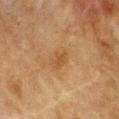notes — catalogued during a skin exam; not biopsied | illumination — cross-polarized | body site — the chest | subject — female, approximately 55 years of age | image source — 15 mm crop, total-body photography | size — ≈2.5 mm | automated lesion analysis — an eccentricity of roughly 0.75 and two-axis asymmetry of about 0.3; a mean CIELAB color near L≈42 a*≈18 b*≈34 and a normalized border contrast of about 5.5; border irregularity of about 3 on a 0–10 scale, a color-variation rating of about 1.5/10, and peripheral color asymmetry of about 0.5.